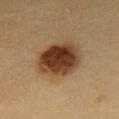{
  "biopsy_status": "not biopsied; imaged during a skin examination",
  "lighting": "cross-polarized",
  "lesion_size": {
    "long_diameter_mm_approx": 5.5
  },
  "patient": {
    "sex": "female",
    "age_approx": 30
  },
  "image": {
    "source": "total-body photography crop",
    "field_of_view_mm": 15
  },
  "site": "chest"
}